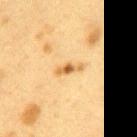No biopsy was performed on this lesion — it was imaged during a full skin examination and was not determined to be concerning. The patient is a female approximately 40 years of age. A 15 mm close-up extracted from a 3D total-body photography capture. From the mid back.